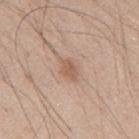<lesion>
  <biopsy_status>not biopsied; imaged during a skin examination</biopsy_status>
  <lesion_size>
    <long_diameter_mm_approx>2.5</long_diameter_mm_approx>
  </lesion_size>
  <lighting>white-light</lighting>
  <site>mid back</site>
  <image>
    <source>total-body photography crop</source>
    <field_of_view_mm>15</field_of_view_mm>
  </image>
  <automated_metrics>
    <cielab_L>58</cielab_L>
    <cielab_a>19</cielab_a>
    <cielab_b>29</cielab_b>
    <vs_skin_darker_L>9.0</vs_skin_darker_L>
    <vs_skin_contrast_norm>6.5</vs_skin_contrast_norm>
    <color_variation_0_10>1.5</color_variation_0_10>
    <peripheral_color_asymmetry>0.5</peripheral_color_asymmetry>
    <nevus_likeness_0_100>45</nevus_likeness_0_100>
    <lesion_detection_confidence_0_100>100</lesion_detection_confidence_0_100>
  </automated_metrics>
  <patient>
    <sex>male</sex>
    <age_approx>50</age_approx>
  </patient>
</lesion>